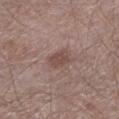Part of a total-body skin-imaging series; this lesion was reviewed on a skin check and was not flagged for biopsy. A male patient, aged 63–67. A close-up tile cropped from a whole-body skin photograph, about 15 mm across. About 3 mm across. The lesion-visualizer software estimated a normalized border contrast of about 6.5. The analysis additionally found a classifier nevus-likeness of about 35/100 and lesion-presence confidence of about 100/100. The lesion is on the left lower leg.